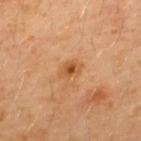Imaged during a routine full-body skin examination; the lesion was not biopsied and no histopathology is available.
The lesion is located on the back.
The total-body-photography lesion software estimated an average lesion color of about L≈52 a*≈24 b*≈41 (CIELAB), a lesion–skin lightness drop of about 10, and a normalized lesion–skin contrast near 7.5. The analysis additionally found border irregularity of about 2.5 on a 0–10 scale, a within-lesion color-variation index near 4/10, and a peripheral color-asymmetry measure near 1. The analysis additionally found a detector confidence of about 100 out of 100 that the crop contains a lesion.
The tile uses cross-polarized illumination.
A 15 mm crop from a total-body photograph taken for skin-cancer surveillance.
Longest diameter approximately 2.5 mm.
The subject is a male aged around 30.